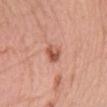Notes:
* workup: no biopsy performed (imaged during a skin exam)
* anatomic site: the chest
* subject: female, approximately 60 years of age
* acquisition: 15 mm crop, total-body photography